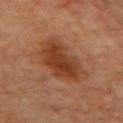<lesion>
  <lesion_size>
    <long_diameter_mm_approx>6.0</long_diameter_mm_approx>
  </lesion_size>
  <image>
    <source>total-body photography crop</source>
    <field_of_view_mm>15</field_of_view_mm>
  </image>
  <automated_metrics>
    <area_mm2_approx>21.0</area_mm2_approx>
    <shape_asymmetry>0.2</shape_asymmetry>
    <nevus_likeness_0_100>60</nevus_likeness_0_100>
    <lesion_detection_confidence_0_100>100</lesion_detection_confidence_0_100>
  </automated_metrics>
  <patient>
    <sex>male</sex>
    <age_approx>80</age_approx>
  </patient>
  <lighting>cross-polarized</lighting>
  <site>mid back</site>
</lesion>